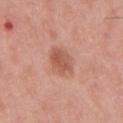Impression:
The lesion was photographed on a routine skin check and not biopsied; there is no pathology result.
Background:
Approximately 3 mm at its widest. Cropped from a total-body skin-imaging series; the visible field is about 15 mm. A male subject aged 53 to 57. Automated image analysis of the tile measured a footprint of about 7 mm², an eccentricity of roughly 0.5, and two-axis asymmetry of about 0.15. The analysis additionally found an average lesion color of about L≈56 a*≈25 b*≈30 (CIELAB), about 10 CIELAB-L* units darker than the surrounding skin, and a normalized lesion–skin contrast near 7. The software also gave a border-irregularity rating of about 2/10, a color-variation rating of about 3/10, and radial color variation of about 1. The lesion is on the mid back. Captured under white-light illumination.